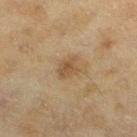Q: Was a biopsy performed?
A: imaged on a skin check; not biopsied
Q: Lesion size?
A: ~2.5 mm (longest diameter)
Q: How was the tile lit?
A: cross-polarized illumination
Q: Who is the patient?
A: female, about 60 years old
Q: Lesion location?
A: the left lower leg
Q: What is the imaging modality?
A: ~15 mm crop, total-body skin-cancer survey